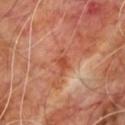Case summary:
- notes: catalogued during a skin exam; not biopsied
- location: the upper back
- acquisition: ~15 mm tile from a whole-body skin photo
- patient: male, aged around 60
- tile lighting: cross-polarized